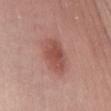The lesion was photographed on a routine skin check and not biopsied; there is no pathology result.
Approximately 4.5 mm at its widest.
The lesion is located on the chest.
Imaged with white-light lighting.
Automated tile analysis of the lesion measured a border-irregularity rating of about 2/10 and internal color variation of about 3 on a 0–10 scale. The software also gave a nevus-likeness score of about 100/100 and a detector confidence of about 100 out of 100 that the crop contains a lesion.
A 15 mm crop from a total-body photograph taken for skin-cancer surveillance.
A female subject roughly 30 years of age.